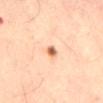| field | value |
|---|---|
| notes | imaged on a skin check; not biopsied |
| subject | male, aged around 65 |
| size | ~2 mm (longest diameter) |
| lighting | cross-polarized illumination |
| imaging modality | 15 mm crop, total-body photography |
| body site | the back |
| automated lesion analysis | a footprint of about 2 mm² and a shape-asymmetry score of about 0.2 (0 = symmetric); an average lesion color of about L≈66 a*≈25 b*≈37 (CIELAB), a lesion–skin lightness drop of about 16, and a normalized lesion–skin contrast near 9.5; a border-irregularity rating of about 1.5/10 and a peripheral color-asymmetry measure near 1.5; a classifier nevus-likeness of about 95/100 |